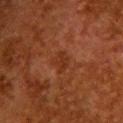Q: Is there a histopathology result?
A: catalogued during a skin exam; not biopsied
Q: How was the tile lit?
A: cross-polarized illumination
Q: What is the lesion's diameter?
A: ~2.5 mm (longest diameter)
Q: How was this image acquired?
A: total-body-photography crop, ~15 mm field of view
Q: Where on the body is the lesion?
A: the upper back
Q: What are the patient's age and sex?
A: female, aged 48–52